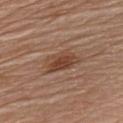notes — imaged on a skin check; not biopsied | body site — the chest | lesion size — ≈4.5 mm | patient — male, aged 78 to 82 | lighting — white-light | acquisition — 15 mm crop, total-body photography | automated lesion analysis — border irregularity of about 3.5 on a 0–10 scale and a peripheral color-asymmetry measure near 1.